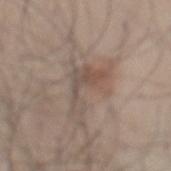| field | value |
|---|---|
| workup | no biopsy performed (imaged during a skin exam) |
| automated lesion analysis | a footprint of about 8.5 mm², an outline eccentricity of about 0.85 (0 = round, 1 = elongated), and two-axis asymmetry of about 0.75 |
| image source | 15 mm crop, total-body photography |
| patient | male, roughly 35 years of age |
| body site | the mid back |
| lesion diameter | ~5 mm (longest diameter) |
| illumination | white-light illumination |